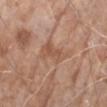Case summary:
• notes · total-body-photography surveillance lesion; no biopsy
• patient · female, in their mid- to late 80s or older
• anatomic site · the right upper arm
• automated metrics · a footprint of about 11 mm² and a symmetry-axis asymmetry near 0.45; an average lesion color of about L≈55 a*≈20 b*≈30 (CIELAB) and a normalized lesion–skin contrast near 5; a border-irregularity rating of about 5/10, a within-lesion color-variation index near 3/10, and a peripheral color-asymmetry measure near 1; an automated nevus-likeness rating near 0 out of 100
• lighting · white-light illumination
• image source · ~15 mm crop, total-body skin-cancer survey
• lesion diameter · ~4.5 mm (longest diameter)